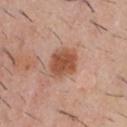The lesion is located on the chest. The recorded lesion diameter is about 4 mm. A 15 mm close-up tile from a total-body photography series done for melanoma screening. The tile uses white-light illumination. The subject is a male aged around 30.